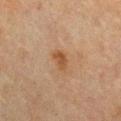automated_metrics:
  vs_skin_darker_L: 7.0
patient:
  sex: female
  age_approx: 60
site: chest
lighting: cross-polarized
lesion_size:
  long_diameter_mm_approx: 3.0
image:
  source: total-body photography crop
  field_of_view_mm: 15